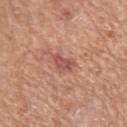biopsy status: catalogued during a skin exam; not biopsied.